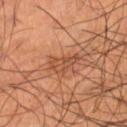| feature | finding |
|---|---|
| follow-up | total-body-photography surveillance lesion; no biopsy |
| location | the right lower leg |
| lighting | cross-polarized illumination |
| size | ~3.5 mm (longest diameter) |
| automated lesion analysis | an automated nevus-likeness rating near 5 out of 100 and a lesion-detection confidence of about 100/100 |
| subject | male, aged approximately 55 |
| imaging modality | total-body-photography crop, ~15 mm field of view |